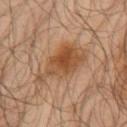Impression: Captured during whole-body skin photography for melanoma surveillance; the lesion was not biopsied. Clinical summary: The lesion is on the left upper arm. A male subject, aged 63–67. Automated image analysis of the tile measured an area of roughly 15 mm², an outline eccentricity of about 0.9 (0 = round, 1 = elongated), and a shape-asymmetry score of about 0.35 (0 = symmetric). The software also gave an automated nevus-likeness rating near 90 out of 100. The tile uses cross-polarized illumination. Approximately 6.5 mm at its widest. Cropped from a whole-body photographic skin survey; the tile spans about 15 mm.